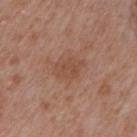Q: Was this lesion biopsied?
A: imaged on a skin check; not biopsied
Q: How was this image acquired?
A: ~15 mm tile from a whole-body skin photo
Q: How large is the lesion?
A: ~3.5 mm (longest diameter)
Q: Who is the patient?
A: male, aged 63 to 67
Q: What lighting was used for the tile?
A: white-light
Q: What is the anatomic site?
A: the mid back